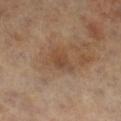{"biopsy_status": "not biopsied; imaged during a skin examination", "lighting": "cross-polarized", "site": "left leg", "lesion_size": {"long_diameter_mm_approx": 3.0}, "automated_metrics": {"area_mm2_approx": 5.5, "eccentricity": 0.7, "shape_asymmetry": 0.25, "nevus_likeness_0_100": 0}, "patient": {"sex": "female", "age_approx": 65}, "image": {"source": "total-body photography crop", "field_of_view_mm": 15}}